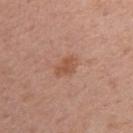This lesion was catalogued during total-body skin photography and was not selected for biopsy.